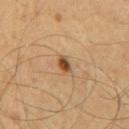Clinical impression: Part of a total-body skin-imaging series; this lesion was reviewed on a skin check and was not flagged for biopsy. Clinical summary: Cropped from a whole-body photographic skin survey; the tile spans about 15 mm. A male patient aged 58 to 62. Located on the mid back.